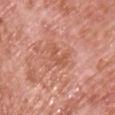  biopsy_status: not biopsied; imaged during a skin examination